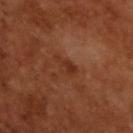follow-up — imaged on a skin check; not biopsied | tile lighting — cross-polarized | imaging modality — 15 mm crop, total-body photography | lesion size — ~3 mm (longest diameter) | patient — male, approximately 65 years of age.